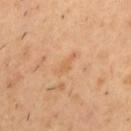No biopsy was performed on this lesion — it was imaged during a full skin examination and was not determined to be concerning. A lesion tile, about 15 mm wide, cut from a 3D total-body photograph. Approximately 3.5 mm at its widest. A male subject in their mid- to late 50s. This is a cross-polarized tile. Located on the upper back. The lesion-visualizer software estimated a lesion area of about 3.5 mm² and a shape eccentricity near 0.9. The analysis additionally found a mean CIELAB color near L≈60 a*≈20 b*≈37, roughly 6 lightness units darker than nearby skin, and a lesion-to-skin contrast of about 4.5 (normalized; higher = more distinct). The analysis additionally found an automated nevus-likeness rating near 0 out of 100.